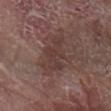The lesion was photographed on a routine skin check and not biopsied; there is no pathology result. Cropped from a total-body skin-imaging series; the visible field is about 15 mm. Captured under white-light illumination. Located on the arm. Measured at roughly 6.5 mm in maximum diameter. A male patient aged 78–82.